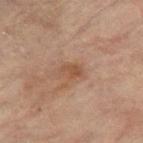<case>
<biopsy_status>not biopsied; imaged during a skin examination</biopsy_status>
<site>left leg</site>
<image>
  <source>total-body photography crop</source>
  <field_of_view_mm>15</field_of_view_mm>
</image>
<patient>
  <sex>female</sex>
  <age_approx>80</age_approx>
</patient>
<lighting>cross-polarized</lighting>
<automated_metrics>
  <cielab_L>46</cielab_L>
  <cielab_a>18</cielab_a>
  <cielab_b>28</cielab_b>
  <vs_skin_darker_L>7.0</vs_skin_darker_L>
  <vs_skin_contrast_norm>6.0</vs_skin_contrast_norm>
  <nevus_likeness_0_100>0</nevus_likeness_0_100>
  <lesion_detection_confidence_0_100>100</lesion_detection_confidence_0_100>
</automated_metrics>
</case>